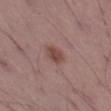Impression:
The lesion was tiled from a total-body skin photograph and was not biopsied.
Context:
A 15 mm close-up tile from a total-body photography series done for melanoma screening. Located on the left thigh. Captured under white-light illumination. The lesion-visualizer software estimated an average lesion color of about L≈46 a*≈20 b*≈23 (CIELAB), a lesion–skin lightness drop of about 9, and a normalized lesion–skin contrast near 7.5. The subject is a male aged approximately 70. Measured at roughly 3 mm in maximum diameter.